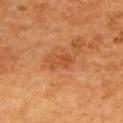Q: Lesion location?
A: the back
Q: What lighting was used for the tile?
A: cross-polarized
Q: Patient demographics?
A: female
Q: How was this image acquired?
A: total-body-photography crop, ~15 mm field of view
Q: What is the lesion's diameter?
A: about 3 mm